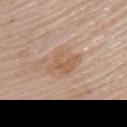biopsy status — imaged on a skin check; not biopsied | TBP lesion metrics — a lesion area of about 7.5 mm², an outline eccentricity of about 0.7 (0 = round, 1 = elongated), and a shape-asymmetry score of about 0.25 (0 = symmetric); an average lesion color of about L≈58 a*≈19 b*≈32 (CIELAB), about 8 CIELAB-L* units darker than the surrounding skin, and a normalized lesion–skin contrast near 6.5 | lesion size — ~3.5 mm (longest diameter) | illumination — white-light | subject — female, roughly 65 years of age | site — the upper back | imaging modality — ~15 mm tile from a whole-body skin photo.